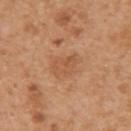Assessment: The lesion was tiled from a total-body skin photograph and was not biopsied. Context: A male subject about 55 years old. From the right upper arm. The total-body-photography lesion software estimated an area of roughly 8.5 mm². The software also gave a lesion color around L≈55 a*≈22 b*≈36 in CIELAB and roughly 7 lightness units darker than nearby skin. And it measured a classifier nevus-likeness of about 0/100 and a lesion-detection confidence of about 100/100. This is a white-light tile. Cropped from a total-body skin-imaging series; the visible field is about 15 mm.